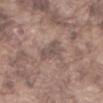  biopsy_status: not biopsied; imaged during a skin examination
  patient:
    sex: male
    age_approx: 75
  automated_metrics:
    area_mm2_approx: 6.5
    eccentricity: 0.7
    cielab_L: 51
    cielab_a: 14
    cielab_b: 19
    vs_skin_darker_L: 8.0
    vs_skin_contrast_norm: 6.5
    border_irregularity_0_10: 5.0
    peripheral_color_asymmetry: 1.0
  lesion_size:
    long_diameter_mm_approx: 3.5
  image:
    source: total-body photography crop
    field_of_view_mm: 15
  site: front of the torso